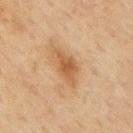follow-up = total-body-photography surveillance lesion; no biopsy
location = the mid back
acquisition = 15 mm crop, total-body photography
subject = male, aged approximately 70